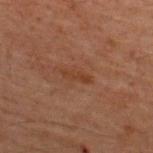{"biopsy_status": "not biopsied; imaged during a skin examination", "image": {"source": "total-body photography crop", "field_of_view_mm": 15}, "patient": {"sex": "male", "age_approx": 60}, "lesion_size": {"long_diameter_mm_approx": 3.0}, "site": "upper back"}